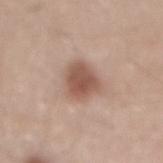Q: Was this lesion biopsied?
A: total-body-photography surveillance lesion; no biopsy
Q: How was the tile lit?
A: white-light
Q: What did automated image analysis measure?
A: a within-lesion color-variation index near 3.5/10 and radial color variation of about 1; an automated nevus-likeness rating near 95 out of 100 and a detector confidence of about 100 out of 100 that the crop contains a lesion
Q: How was this image acquired?
A: 15 mm crop, total-body photography
Q: Where on the body is the lesion?
A: the mid back
Q: Who is the patient?
A: male, roughly 45 years of age
Q: Lesion size?
A: ≈4 mm